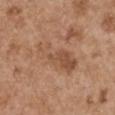Part of a total-body skin-imaging series; this lesion was reviewed on a skin check and was not flagged for biopsy.
The lesion is located on the left upper arm.
The patient is a male approximately 55 years of age.
Automated tile analysis of the lesion measured a normalized lesion–skin contrast near 5.5. The analysis additionally found a border-irregularity rating of about 4.5/10 and peripheral color asymmetry of about 2.
Cropped from a whole-body photographic skin survey; the tile spans about 15 mm.
Imaged with white-light lighting.